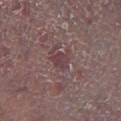Captured during whole-body skin photography for melanoma surveillance; the lesion was not biopsied.
A male patient approximately 80 years of age.
A close-up tile cropped from a whole-body skin photograph, about 15 mm across.
Imaged with white-light lighting.
Measured at roughly 2.5 mm in maximum diameter.
The lesion is on the leg.